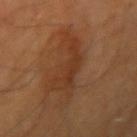follow-up: total-body-photography surveillance lesion; no biopsy | acquisition: 15 mm crop, total-body photography | patient: male | anatomic site: the right upper arm.